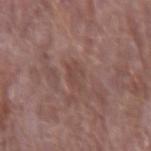No biopsy was performed on this lesion — it was imaged during a full skin examination and was not determined to be concerning. A region of skin cropped from a whole-body photographic capture, roughly 15 mm wide. Measured at roughly 3 mm in maximum diameter. From the right forearm. A male patient in their mid- to late 60s. This is a white-light tile.